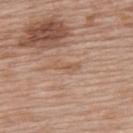{"patient": {"sex": "female", "age_approx": 60}, "site": "upper back", "image": {"source": "total-body photography crop", "field_of_view_mm": 15}}